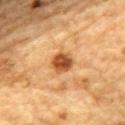Assessment: This lesion was catalogued during total-body skin photography and was not selected for biopsy. Acquisition and patient details: Located on the mid back. A close-up tile cropped from a whole-body skin photograph, about 15 mm across. The patient is a male about 85 years old or older.